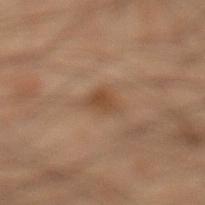Impression:
The lesion was tiled from a total-body skin photograph and was not biopsied.
Background:
A close-up tile cropped from a whole-body skin photograph, about 15 mm across. From the leg. The patient is a male roughly 50 years of age.